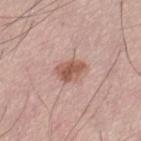A 15 mm close-up extracted from a 3D total-body photography capture.
A male patient, approximately 70 years of age.
On the left thigh.